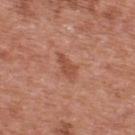<record>
  <biopsy_status>not biopsied; imaged during a skin examination</biopsy_status>
  <lighting>white-light</lighting>
  <patient>
    <sex>male</sex>
    <age_approx>70</age_approx>
  </patient>
  <site>upper back</site>
  <image>
    <source>total-body photography crop</source>
    <field_of_view_mm>15</field_of_view_mm>
  </image>
  <lesion_size>
    <long_diameter_mm_approx>3.5</long_diameter_mm_approx>
  </lesion_size>
</record>